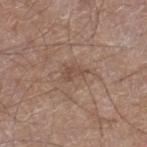Clinical impression: Imaged during a routine full-body skin examination; the lesion was not biopsied and no histopathology is available. Context: A male subject in their 60s. From the leg. The total-body-photography lesion software estimated a lesion area of about 3 mm², an eccentricity of roughly 0.85, and a symmetry-axis asymmetry near 0.45. It also reported a lesion color around L≈48 a*≈17 b*≈25 in CIELAB, about 8 CIELAB-L* units darker than the surrounding skin, and a lesion-to-skin contrast of about 6 (normalized; higher = more distinct). The software also gave an automated nevus-likeness rating near 0 out of 100 and a detector confidence of about 100 out of 100 that the crop contains a lesion. Longest diameter approximately 2.5 mm. A 15 mm close-up extracted from a 3D total-body photography capture. This is a white-light tile.